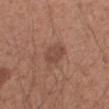biopsy_status: not biopsied; imaged during a skin examination
lighting: white-light
image:
  source: total-body photography crop
  field_of_view_mm: 15
lesion_size:
  long_diameter_mm_approx: 3.0
patient:
  sex: male
  age_approx: 50
automated_metrics:
  area_mm2_approx: 6.0
  eccentricity: 0.55
  shape_asymmetry: 0.2
  border_irregularity_0_10: 2.0
  peripheral_color_asymmetry: 1.0
site: right forearm